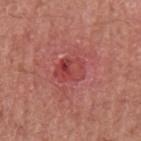Assessment:
This lesion was catalogued during total-body skin photography and was not selected for biopsy.
Background:
Approximately 3.5 mm at its widest. A 15 mm close-up extracted from a 3D total-body photography capture. From the arm. The patient is a male aged approximately 45. Captured under white-light illumination.